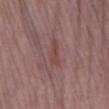* biopsy status: imaged on a skin check; not biopsied
* automated metrics: an area of roughly 3 mm², an eccentricity of roughly 0.95, and two-axis asymmetry of about 0.45; a lesion-detection confidence of about 50/100
* body site: the left lower leg
* image source: total-body-photography crop, ~15 mm field of view
* lesion diameter: ~3.5 mm (longest diameter)
* illumination: white-light
* subject: female, roughly 65 years of age